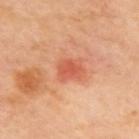Clinical impression:
No biopsy was performed on this lesion — it was imaged during a full skin examination and was not determined to be concerning.
Image and clinical context:
On the upper back. This image is a 15 mm lesion crop taken from a total-body photograph. The lesion's longest dimension is about 3 mm.